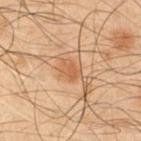image: ~15 mm tile from a whole-body skin photo | location: the arm | subject: male, approximately 50 years of age.